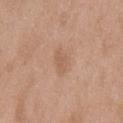notes = total-body-photography surveillance lesion; no biopsy.